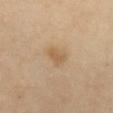No biopsy was performed on this lesion — it was imaged during a full skin examination and was not determined to be concerning. The subject is a female aged around 60. The lesion's longest dimension is about 2.5 mm. Automated image analysis of the tile measured a lesion area of about 3 mm², an eccentricity of roughly 0.8, and a symmetry-axis asymmetry near 0.3. The software also gave an average lesion color of about L≈58 a*≈16 b*≈37 (CIELAB) and a normalized border contrast of about 6.5. The lesion is located on the chest. Cropped from a total-body skin-imaging series; the visible field is about 15 mm. Captured under cross-polarized illumination.